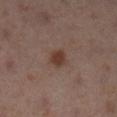{
  "image": {
    "source": "total-body photography crop",
    "field_of_view_mm": 15
  },
  "lesion_size": {
    "long_diameter_mm_approx": 2.5
  },
  "patient": {
    "sex": "female",
    "age_approx": 40
  },
  "site": "left lower leg"
}